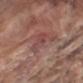The lesion was tiled from a total-body skin photograph and was not biopsied. A roughly 15 mm field-of-view crop from a total-body skin photograph. Captured under white-light illumination. A male subject in their mid- to late 70s. The total-body-photography lesion software estimated an eccentricity of roughly 0.9 and a symmetry-axis asymmetry near 0.55. And it measured roughly 7 lightness units darker than nearby skin. The software also gave border irregularity of about 7 on a 0–10 scale and a peripheral color-asymmetry measure near 0.5. On the left upper arm.